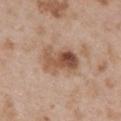follow-up — total-body-photography surveillance lesion; no biopsy
anatomic site — the upper back
image — ~15 mm crop, total-body skin-cancer survey
subject — female, roughly 30 years of age